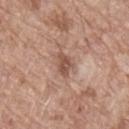Q: Was a biopsy performed?
A: no biopsy performed (imaged during a skin exam)
Q: Patient demographics?
A: male, in their 80s
Q: What kind of image is this?
A: ~15 mm crop, total-body skin-cancer survey
Q: Lesion size?
A: ≈3 mm
Q: What is the anatomic site?
A: the lower back
Q: How was the tile lit?
A: white-light illumination